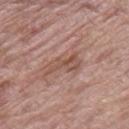{
  "biopsy_status": "not biopsied; imaged during a skin examination",
  "image": {
    "source": "total-body photography crop",
    "field_of_view_mm": 15
  },
  "patient": {
    "sex": "female",
    "age_approx": 70
  },
  "site": "right thigh"
}